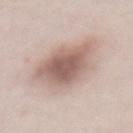notes = catalogued during a skin exam; not biopsied
site = the abdomen
image-analysis metrics = a mean CIELAB color near L≈61 a*≈17 b*≈23, about 13 CIELAB-L* units darker than the surrounding skin, and a normalized border contrast of about 8; a detector confidence of about 100 out of 100 that the crop contains a lesion
subject = female, in their 40s
illumination = white-light illumination
image = 15 mm crop, total-body photography